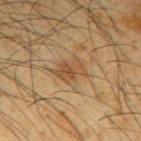Q: Was a biopsy performed?
A: catalogued during a skin exam; not biopsied
Q: Who is the patient?
A: male, aged 33–37
Q: What did automated image analysis measure?
A: a lesion area of about 7.5 mm² and an outline eccentricity of about 0.6 (0 = round, 1 = elongated); a mean CIELAB color near L≈38 a*≈14 b*≈28, a lesion–skin lightness drop of about 7, and a normalized lesion–skin contrast near 6; a border-irregularity rating of about 3.5/10, a color-variation rating of about 4/10, and peripheral color asymmetry of about 1; an automated nevus-likeness rating near 45 out of 100 and lesion-presence confidence of about 95/100
Q: What kind of image is this?
A: 15 mm crop, total-body photography
Q: Where on the body is the lesion?
A: the right upper arm
Q: How was the tile lit?
A: cross-polarized illumination
Q: What is the lesion's diameter?
A: ≈3.5 mm